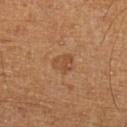Impression:
Part of a total-body skin-imaging series; this lesion was reviewed on a skin check and was not flagged for biopsy.
Image and clinical context:
Located on the leg. The subject is a male roughly 60 years of age. A close-up tile cropped from a whole-body skin photograph, about 15 mm across.